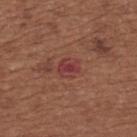| feature | finding |
|---|---|
| lesion diameter | ~2.5 mm (longest diameter) |
| patient | female, aged 63 to 67 |
| anatomic site | the upper back |
| lighting | white-light |
| automated lesion analysis | an area of roughly 5 mm², an outline eccentricity of about 0.45 (0 = round, 1 = elongated), and a shape-asymmetry score of about 0.2 (0 = symmetric); a lesion color around L≈39 a*≈29 b*≈23 in CIELAB, about 7 CIELAB-L* units darker than the surrounding skin, and a normalized lesion–skin contrast near 7 |
| image source | 15 mm crop, total-body photography |